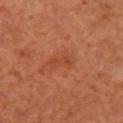{"patient": {"sex": "female", "age_approx": 55}, "lesion_size": {"long_diameter_mm_approx": 2.5}, "automated_metrics": {"eccentricity": 0.9, "border_irregularity_0_10": 5.0, "color_variation_0_10": 0.0, "peripheral_color_asymmetry": 0.0, "nevus_likeness_0_100": 0, "lesion_detection_confidence_0_100": 100}, "lighting": "cross-polarized", "image": {"source": "total-body photography crop", "field_of_view_mm": 15}, "site": "right upper arm"}